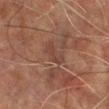{
  "biopsy_status": "not biopsied; imaged during a skin examination",
  "image": {
    "source": "total-body photography crop",
    "field_of_view_mm": 15
  },
  "lighting": "cross-polarized",
  "site": "leg",
  "lesion_size": {
    "long_diameter_mm_approx": 3.0
  },
  "patient": {
    "sex": "male",
    "age_approx": 70
  }
}